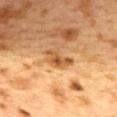Clinical impression:
Part of a total-body skin-imaging series; this lesion was reviewed on a skin check and was not flagged for biopsy.
Background:
The subject is a female about 40 years old. A roughly 15 mm field-of-view crop from a total-body skin photograph. An algorithmic analysis of the crop reported a footprint of about 5 mm². The analysis additionally found a mean CIELAB color near L≈46 a*≈20 b*≈37, roughly 10 lightness units darker than nearby skin, and a normalized border contrast of about 8. And it measured border irregularity of about 5.5 on a 0–10 scale and internal color variation of about 3 on a 0–10 scale. Imaged with cross-polarized lighting. Located on the upper back.